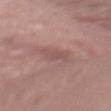{
  "biopsy_status": "not biopsied; imaged during a skin examination",
  "patient": {
    "sex": "female",
    "age_approx": 50
  },
  "site": "left lower leg",
  "image": {
    "source": "total-body photography crop",
    "field_of_view_mm": 15
  }
}